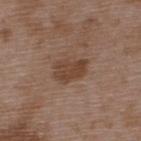{"biopsy_status": "not biopsied; imaged during a skin examination", "lesion_size": {"long_diameter_mm_approx": 4.0}, "site": "upper back", "patient": {"sex": "male", "age_approx": 50}, "image": {"source": "total-body photography crop", "field_of_view_mm": 15}, "lighting": "white-light"}